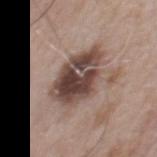Findings:
* biopsy status — imaged on a skin check; not biopsied
* anatomic site — the front of the torso
* automated lesion analysis — a normalized lesion–skin contrast near 11.5; a border-irregularity index near 2.5/10 and a color-variation rating of about 9/10
* lesion diameter — ≈6.5 mm
* patient — male, approximately 65 years of age
* image source — 15 mm crop, total-body photography